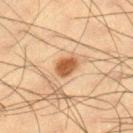Impression: The lesion was photographed on a routine skin check and not biopsied; there is no pathology result. Background: A male subject, in their mid- to late 30s. Measured at roughly 2.5 mm in maximum diameter. A 15 mm close-up tile from a total-body photography series done for melanoma screening. The lesion is on the right lower leg. Captured under cross-polarized illumination.